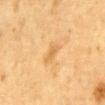{
  "biopsy_status": "not biopsied; imaged during a skin examination",
  "lesion_size": {
    "long_diameter_mm_approx": 2.5
  },
  "lighting": "cross-polarized",
  "patient": {
    "sex": "male",
    "age_approx": "85+"
  },
  "site": "abdomen",
  "image": {
    "source": "total-body photography crop",
    "field_of_view_mm": 15
  }
}